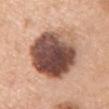Case summary:
– notes: total-body-photography surveillance lesion; no biopsy
– lighting: white-light illumination
– image source: total-body-photography crop, ~15 mm field of view
– body site: the left upper arm
– subject: female, aged 58 to 62